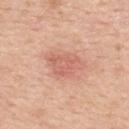Imaged during a routine full-body skin examination; the lesion was not biopsied and no histopathology is available. A 15 mm close-up tile from a total-body photography series done for melanoma screening. About 4.5 mm across. This is a white-light tile. The subject is a male approximately 50 years of age. The lesion is located on the mid back.